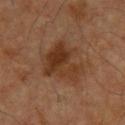Recorded during total-body skin imaging; not selected for excision or biopsy.
A roughly 15 mm field-of-view crop from a total-body skin photograph.
The lesion is located on the chest.
A male patient approximately 60 years of age.